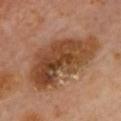Assessment: Captured during whole-body skin photography for melanoma surveillance; the lesion was not biopsied. Background: The lesion is on the chest. Captured under cross-polarized illumination. The patient is a female aged approximately 60. The lesion's longest dimension is about 10 mm. A roughly 15 mm field-of-view crop from a total-body skin photograph.